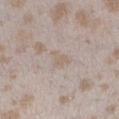workup: imaged on a skin check; not biopsied
automated lesion analysis: a footprint of about 3.5 mm², an eccentricity of roughly 0.65, and two-axis asymmetry of about 0.5; a mean CIELAB color near L≈61 a*≈11 b*≈23, roughly 5 lightness units darker than nearby skin, and a lesion-to-skin contrast of about 4.5 (normalized; higher = more distinct); a nevus-likeness score of about 0/100 and a lesion-detection confidence of about 60/100
imaging modality: ~15 mm crop, total-body skin-cancer survey
body site: the leg
patient: female, aged around 25